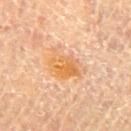Clinical impression:
This lesion was catalogued during total-body skin photography and was not selected for biopsy.
Context:
The lesion is on the chest. The recorded lesion diameter is about 4.5 mm. This image is a 15 mm lesion crop taken from a total-body photograph. The tile uses cross-polarized illumination. A male patient about 75 years old.